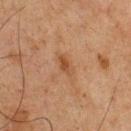Impression:
Imaged during a routine full-body skin examination; the lesion was not biopsied and no histopathology is available.
Background:
A male subject aged approximately 75. Measured at roughly 3 mm in maximum diameter. The lesion is located on the chest. A 15 mm crop from a total-body photograph taken for skin-cancer surveillance. Imaged with cross-polarized lighting.